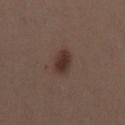Assessment: Part of a total-body skin-imaging series; this lesion was reviewed on a skin check and was not flagged for biopsy. Context: The lesion-visualizer software estimated an area of roughly 5.5 mm², a shape eccentricity near 0.75, and a shape-asymmetry score of about 0.15 (0 = symmetric). It also reported border irregularity of about 1.5 on a 0–10 scale, a color-variation rating of about 2/10, and peripheral color asymmetry of about 0.5. The software also gave a classifier nevus-likeness of about 100/100. A female subject, aged approximately 40. Imaged with white-light lighting. A 15 mm crop from a total-body photograph taken for skin-cancer surveillance. The recorded lesion diameter is about 3 mm. Located on the left thigh.